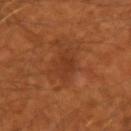Part of a total-body skin-imaging series; this lesion was reviewed on a skin check and was not flagged for biopsy.
The patient is a male aged 48 to 52.
An algorithmic analysis of the crop reported a mean CIELAB color near L≈36 a*≈26 b*≈34, roughly 6 lightness units darker than nearby skin, and a lesion-to-skin contrast of about 5.5 (normalized; higher = more distinct). The software also gave lesion-presence confidence of about 100/100.
Cropped from a whole-body photographic skin survey; the tile spans about 15 mm.
The lesion is located on the left upper arm.
The tile uses cross-polarized illumination.